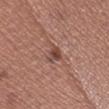Impression: This lesion was catalogued during total-body skin photography and was not selected for biopsy.